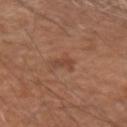Clinical impression: Recorded during total-body skin imaging; not selected for excision or biopsy. Background: The patient is a male aged 68–72. The lesion's longest dimension is about 2.5 mm. Located on the right upper arm. A lesion tile, about 15 mm wide, cut from a 3D total-body photograph. An algorithmic analysis of the crop reported a mean CIELAB color near L≈44 a*≈22 b*≈30, a lesion–skin lightness drop of about 7, and a normalized lesion–skin contrast near 5.5. It also reported a color-variation rating of about 1/10 and peripheral color asymmetry of about 0. And it measured lesion-presence confidence of about 100/100. Captured under white-light illumination.